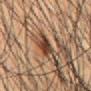Assessment: Captured during whole-body skin photography for melanoma surveillance; the lesion was not biopsied. Acquisition and patient details: Cropped from a whole-body photographic skin survey; the tile spans about 15 mm. Automated tile analysis of the lesion measured a lesion area of about 5.5 mm², an eccentricity of roughly 0.45, and two-axis asymmetry of about 0.25. The analysis additionally found a mean CIELAB color near L≈39 a*≈19 b*≈29 and a lesion-to-skin contrast of about 10.5 (normalized; higher = more distinct). The software also gave a border-irregularity rating of about 3/10, internal color variation of about 6.5 on a 0–10 scale, and a peripheral color-asymmetry measure near 2.5. The analysis additionally found a nevus-likeness score of about 50/100 and lesion-presence confidence of about 70/100. A male patient aged 48–52. The lesion is located on the abdomen. About 3 mm across. This is a cross-polarized tile.